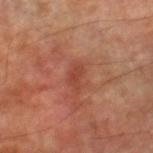Recorded during total-body skin imaging; not selected for excision or biopsy.
Captured under cross-polarized illumination.
The recorded lesion diameter is about 3 mm.
From the right lower leg.
A lesion tile, about 15 mm wide, cut from a 3D total-body photograph.
A male subject about 70 years old.